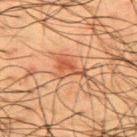Q: Where on the body is the lesion?
A: the upper back
Q: How was this image acquired?
A: ~15 mm tile from a whole-body skin photo
Q: Patient demographics?
A: male, aged 58 to 62